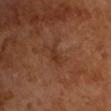Recorded during total-body skin imaging; not selected for excision or biopsy. The subject is a male aged 63–67. Captured under cross-polarized illumination. A region of skin cropped from a whole-body photographic capture, roughly 15 mm wide. The total-body-photography lesion software estimated an area of roughly 3.5 mm² and a shape eccentricity near 0.85. And it measured a mean CIELAB color near L≈33 a*≈22 b*≈30, about 6 CIELAB-L* units darker than the surrounding skin, and a lesion-to-skin contrast of about 5.5 (normalized; higher = more distinct). The analysis additionally found a border-irregularity rating of about 4.5/10, a color-variation rating of about 1/10, and peripheral color asymmetry of about 0.5. And it measured an automated nevus-likeness rating near 0 out of 100 and a lesion-detection confidence of about 100/100. The lesion's longest dimension is about 2.5 mm.